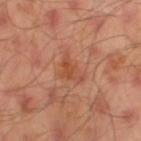Impression:
No biopsy was performed on this lesion — it was imaged during a full skin examination and was not determined to be concerning.
Clinical summary:
The lesion is located on the left thigh. A roughly 15 mm field-of-view crop from a total-body skin photograph. A male subject, aged approximately 45.